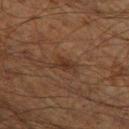Q: Is there a histopathology result?
A: imaged on a skin check; not biopsied
Q: Who is the patient?
A: male, in their 60s
Q: What kind of image is this?
A: ~15 mm tile from a whole-body skin photo
Q: How was the tile lit?
A: cross-polarized illumination
Q: Automated lesion metrics?
A: an area of roughly 4 mm², an outline eccentricity of about 0.8 (0 = round, 1 = elongated), and a symmetry-axis asymmetry near 0.3; a mean CIELAB color near L≈27 a*≈15 b*≈23 and a lesion-to-skin contrast of about 6.5 (normalized; higher = more distinct); a nevus-likeness score of about 50/100 and a detector confidence of about 100 out of 100 that the crop contains a lesion
Q: Where on the body is the lesion?
A: the left thigh
Q: Lesion size?
A: ≈3 mm